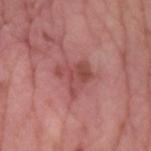Impression: Part of a total-body skin-imaging series; this lesion was reviewed on a skin check and was not flagged for biopsy. Background: Approximately 3.5 mm at its widest. From the arm. A 15 mm close-up extracted from a 3D total-body photography capture. Automated image analysis of the tile measured an area of roughly 8.5 mm², a shape eccentricity near 0.4, and a shape-asymmetry score of about 0.5 (0 = symmetric). The software also gave border irregularity of about 5 on a 0–10 scale, a within-lesion color-variation index near 4/10, and peripheral color asymmetry of about 1. This is a white-light tile. A male subject, in their mid-50s.